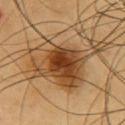Captured during whole-body skin photography for melanoma surveillance; the lesion was not biopsied. The recorded lesion diameter is about 9.5 mm. A lesion tile, about 15 mm wide, cut from a 3D total-body photograph. The lesion-visualizer software estimated a lesion color around L≈46 a*≈20 b*≈37 in CIELAB and about 14 CIELAB-L* units darker than the surrounding skin. The software also gave a nevus-likeness score of about 95/100 and a lesion-detection confidence of about 100/100. The subject is a male in their 60s. Imaged with cross-polarized lighting. From the chest.